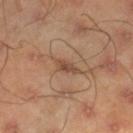Case summary:
- anatomic site · the right lower leg
- patient · male, in their mid-40s
- imaging modality · total-body-photography crop, ~15 mm field of view
- lesion size · ≈3 mm
- illumination · cross-polarized